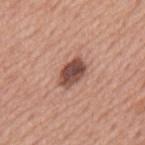Case summary:
• notes: total-body-photography surveillance lesion; no biopsy
• automated lesion analysis: a footprint of about 7 mm², an outline eccentricity of about 0.8 (0 = round, 1 = elongated), and a shape-asymmetry score of about 0.2 (0 = symmetric); border irregularity of about 2 on a 0–10 scale, internal color variation of about 5 on a 0–10 scale, and peripheral color asymmetry of about 1.5
• image source: total-body-photography crop, ~15 mm field of view
• lighting: white-light illumination
• site: the mid back
• subject: male, aged around 75
• lesion size: about 3.5 mm